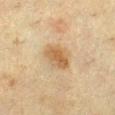Notes:
• follow-up: no biopsy performed (imaged during a skin exam)
• subject: female, aged 53 to 57
• imaging modality: 15 mm crop, total-body photography
• lighting: cross-polarized illumination
• lesion size: ≈3.5 mm
• automated lesion analysis: a shape eccentricity near 0.6 and a shape-asymmetry score of about 0.2 (0 = symmetric); a mean CIELAB color near L≈49 a*≈14 b*≈33, about 9 CIELAB-L* units darker than the surrounding skin, and a normalized border contrast of about 7.5; a border-irregularity index near 1.5/10 and a peripheral color-asymmetry measure near 1.5; a classifier nevus-likeness of about 80/100
• site: the left lower leg